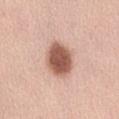Assessment:
This lesion was catalogued during total-body skin photography and was not selected for biopsy.
Context:
The patient is a female aged 58 to 62. The lesion is on the abdomen. A roughly 15 mm field-of-view crop from a total-body skin photograph. Captured under white-light illumination. An algorithmic analysis of the crop reported an average lesion color of about L≈56 a*≈23 b*≈28 (CIELAB) and roughly 18 lightness units darker than nearby skin. The analysis additionally found a classifier nevus-likeness of about 100/100 and a lesion-detection confidence of about 100/100.